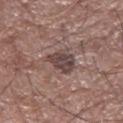Recorded during total-body skin imaging; not selected for excision or biopsy.